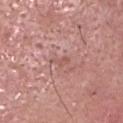• notes — imaged on a skin check; not biopsied
• image — total-body-photography crop, ~15 mm field of view
• anatomic site — the head or neck
• subject — male, approximately 60 years of age
• lesion diameter — ≈2.5 mm